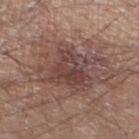Captured during whole-body skin photography for melanoma surveillance; the lesion was not biopsied.